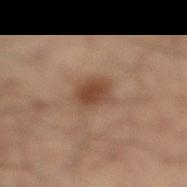Q: Was a biopsy performed?
A: imaged on a skin check; not biopsied
Q: Lesion location?
A: the left leg
Q: What did automated image analysis measure?
A: a shape eccentricity near 0.65 and two-axis asymmetry of about 0.2; a within-lesion color-variation index near 3/10 and peripheral color asymmetry of about 1
Q: Patient demographics?
A: male, about 50 years old
Q: What kind of image is this?
A: 15 mm crop, total-body photography